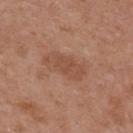Notes:
• workup — total-body-photography surveillance lesion; no biopsy
• acquisition — ~15 mm tile from a whole-body skin photo
• subject — female, aged 38–42
• anatomic site — the upper back